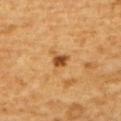Recorded during total-body skin imaging; not selected for excision or biopsy. The subject is a male aged 58–62. From the upper back. Captured under cross-polarized illumination. The recorded lesion diameter is about 2 mm. A 15 mm crop from a total-body photograph taken for skin-cancer surveillance. The lesion-visualizer software estimated internal color variation of about 2.5 on a 0–10 scale and peripheral color asymmetry of about 1. The analysis additionally found lesion-presence confidence of about 100/100.